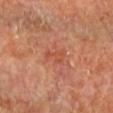The lesion is on the right lower leg. A 15 mm close-up tile from a total-body photography series done for melanoma screening. A male subject about 65 years old. The lesion's longest dimension is about 3 mm. Captured under cross-polarized illumination.